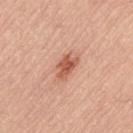Background:
This is a white-light tile. Approximately 3.5 mm at its widest. The subject is a male aged 63 to 67. On the lower back. A 15 mm close-up tile from a total-body photography series done for melanoma screening.